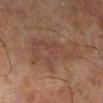Q: Is there a histopathology result?
A: imaged on a skin check; not biopsied
Q: What lighting was used for the tile?
A: cross-polarized illumination
Q: What is the lesion's diameter?
A: about 8 mm
Q: Lesion location?
A: the right lower leg
Q: What kind of image is this?
A: ~15 mm crop, total-body skin-cancer survey
Q: Who is the patient?
A: male, approximately 70 years of age